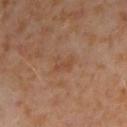workup — catalogued during a skin exam; not biopsied
lighting — cross-polarized illumination
location — the arm
patient — female, approximately 50 years of age
imaging modality — 15 mm crop, total-body photography